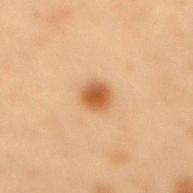{
  "biopsy_status": "not biopsied; imaged during a skin examination",
  "image": {
    "source": "total-body photography crop",
    "field_of_view_mm": 15
  },
  "site": "back",
  "automated_metrics": {
    "nevus_likeness_0_100": 100,
    "lesion_detection_confidence_0_100": 100
  },
  "lighting": "cross-polarized",
  "patient": {
    "sex": "male",
    "age_approx": 55
  }
}